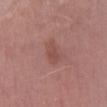Q: Was this lesion biopsied?
A: no biopsy performed (imaged during a skin exam)
Q: Patient demographics?
A: female, aged approximately 60
Q: How large is the lesion?
A: about 3 mm
Q: Illumination type?
A: white-light
Q: What is the imaging modality?
A: ~15 mm tile from a whole-body skin photo
Q: Where on the body is the lesion?
A: the leg